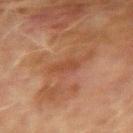The lesion was tiled from a total-body skin photograph and was not biopsied.
The lesion-visualizer software estimated an area of roughly 3.5 mm², a shape eccentricity near 0.85, and a symmetry-axis asymmetry near 0.25. The analysis additionally found a within-lesion color-variation index near 0.5/10 and radial color variation of about 0.
A lesion tile, about 15 mm wide, cut from a 3D total-body photograph.
Longest diameter approximately 3 mm.
This is a cross-polarized tile.
From the left upper arm.
A male patient, in their 70s.